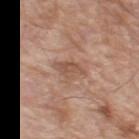This lesion was catalogued during total-body skin photography and was not selected for biopsy.
A male patient roughly 80 years of age.
Automated image analysis of the tile measured a footprint of about 5 mm², an eccentricity of roughly 0.8, and a symmetry-axis asymmetry near 0.3. It also reported a mean CIELAB color near L≈53 a*≈20 b*≈28, a lesion–skin lightness drop of about 9, and a normalized border contrast of about 6. The analysis additionally found a border-irregularity index near 3.5/10 and radial color variation of about 0.5.
Located on the left thigh.
This is a white-light tile.
A region of skin cropped from a whole-body photographic capture, roughly 15 mm wide.
Longest diameter approximately 3.5 mm.